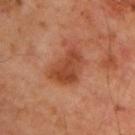Measured at roughly 4.5 mm in maximum diameter.
A male subject roughly 65 years of age.
A close-up tile cropped from a whole-body skin photograph, about 15 mm across.
The lesion is on the upper back.